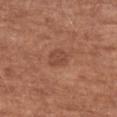• biopsy status — catalogued during a skin exam; not biopsied
• body site — the right upper arm
• acquisition — ~15 mm crop, total-body skin-cancer survey
• diameter — ~2.5 mm (longest diameter)
• automated metrics — a color-variation rating of about 1.5/10 and peripheral color asymmetry of about 0.5
• lighting — white-light illumination
• patient — male, roughly 85 years of age or older